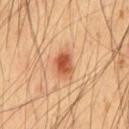Clinical impression: Recorded during total-body skin imaging; not selected for excision or biopsy. Clinical summary: The lesion-visualizer software estimated a border-irregularity index near 2/10, internal color variation of about 3.5 on a 0–10 scale, and a peripheral color-asymmetry measure near 1. And it measured a classifier nevus-likeness of about 100/100 and lesion-presence confidence of about 100/100. Longest diameter approximately 3 mm. A male subject aged around 55. The lesion is on the chest. A lesion tile, about 15 mm wide, cut from a 3D total-body photograph. The tile uses cross-polarized illumination.